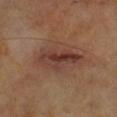Q: Is there a histopathology result?
A: total-body-photography surveillance lesion; no biopsy
Q: What is the anatomic site?
A: the left leg
Q: What is the lesion's diameter?
A: ≈6 mm
Q: How was this image acquired?
A: total-body-photography crop, ~15 mm field of view
Q: Who is the patient?
A: male, in their mid- to late 60s
Q: What lighting was used for the tile?
A: cross-polarized illumination